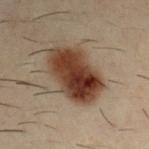Measured at roughly 7.5 mm in maximum diameter. Cropped from a whole-body photographic skin survey; the tile spans about 15 mm. Located on the right upper arm. The patient is a male aged 28 to 32. Captured under cross-polarized illumination. On biopsy, histopathology showed a dysplastic (Clark) nevus.